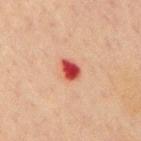Q: Is there a histopathology result?
A: catalogued during a skin exam; not biopsied
Q: What is the anatomic site?
A: the chest
Q: What are the patient's age and sex?
A: male, about 70 years old
Q: What is the imaging modality?
A: total-body-photography crop, ~15 mm field of view